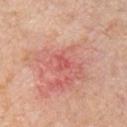Notes:
* automated lesion analysis — an area of roughly 1.5 mm², a shape eccentricity near 0.65, and a symmetry-axis asymmetry near 0.45; a lesion-to-skin contrast of about 4.5 (normalized; higher = more distinct); a nevus-likeness score of about 0/100 and a lesion-detection confidence of about 100/100
* location — the chest
* lighting — white-light
* subject — male, about 60 years old
* lesion size — ~1.5 mm (longest diameter)
* image source — ~15 mm crop, total-body skin-cancer survey
* histopathologic diagnosis — a superficial basal cell carcinoma (malignant)